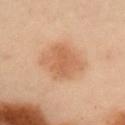Imaged during a routine full-body skin examination; the lesion was not biopsied and no histopathology is available.
This is a cross-polarized tile.
Located on the right upper arm.
A 15 mm crop from a total-body photograph taken for skin-cancer surveillance.
The patient is a male aged around 55.
Longest diameter approximately 4.5 mm.